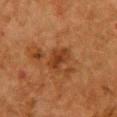workup: catalogued during a skin exam; not biopsied
patient: female, aged 48–52
imaging modality: ~15 mm tile from a whole-body skin photo
body site: the chest
size: ≈3 mm